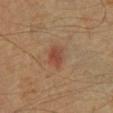A male subject in their 60s.
Located on the back.
This is a cross-polarized tile.
A 15 mm close-up extracted from a 3D total-body photography capture.
About 3.5 mm across.